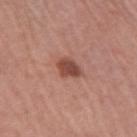Findings:
• workup — total-body-photography surveillance lesion; no biopsy
• automated metrics — roughly 13 lightness units darker than nearby skin; a border-irregularity rating of about 2/10, a color-variation rating of about 2.5/10, and a peripheral color-asymmetry measure near 1; a nevus-likeness score of about 85/100 and lesion-presence confidence of about 100/100
• lesion diameter — about 2.5 mm
• illumination — white-light illumination
• body site — the right upper arm
• imaging modality — total-body-photography crop, ~15 mm field of view
• subject — female, about 65 years old